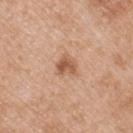No biopsy was performed on this lesion — it was imaged during a full skin examination and was not determined to be concerning.